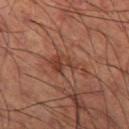Clinical impression: Recorded during total-body skin imaging; not selected for excision or biopsy. Image and clinical context: A male subject, aged 58–62. Located on the left thigh. A 15 mm close-up tile from a total-body photography series done for melanoma screening.